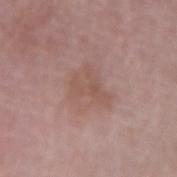Captured during whole-body skin photography for melanoma surveillance; the lesion was not biopsied.
The patient is a female in their 60s.
The lesion-visualizer software estimated two-axis asymmetry of about 0.3. It also reported an average lesion color of about L≈54 a*≈19 b*≈24 (CIELAB), about 6 CIELAB-L* units darker than the surrounding skin, and a normalized border contrast of about 5.
A 15 mm close-up extracted from a 3D total-body photography capture.
The tile uses white-light illumination.
About 4.5 mm across.
The lesion is located on the right forearm.